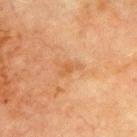Imaged during a routine full-body skin examination; the lesion was not biopsied and no histopathology is available. The tile uses cross-polarized illumination. A male patient, aged around 70. From the front of the torso. A region of skin cropped from a whole-body photographic capture, roughly 15 mm wide. Measured at roughly 2.5 mm in maximum diameter.